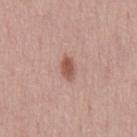Q: Is there a histopathology result?
A: no biopsy performed (imaged during a skin exam)
Q: How was this image acquired?
A: total-body-photography crop, ~15 mm field of view
Q: What is the anatomic site?
A: the chest
Q: Who is the patient?
A: male, aged 43–47
Q: How large is the lesion?
A: about 2.5 mm
Q: What lighting was used for the tile?
A: white-light